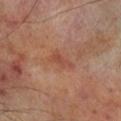biopsy_status: not biopsied; imaged during a skin examination